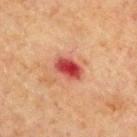The lesion is on the front of the torso. About 3.5 mm across. Automated tile analysis of the lesion measured an average lesion color of about L≈43 a*≈35 b*≈28 (CIELAB). The analysis additionally found a nevus-likeness score of about 0/100. This is a cross-polarized tile. The patient is a male aged around 65. A lesion tile, about 15 mm wide, cut from a 3D total-body photograph.